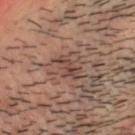biopsy status — total-body-photography surveillance lesion; no biopsy | image — ~15 mm crop, total-body skin-cancer survey | anatomic site — the head or neck | subject — male, aged 38–42 | size — ≈4 mm | illumination — cross-polarized.